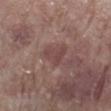The lesion was tiled from a total-body skin photograph and was not biopsied.
This is a white-light tile.
Approximately 3 mm at its widest.
An algorithmic analysis of the crop reported a footprint of about 5 mm², an eccentricity of roughly 0.45, and two-axis asymmetry of about 0.55. And it measured a border-irregularity rating of about 5/10, internal color variation of about 2 on a 0–10 scale, and a peripheral color-asymmetry measure near 0.5. It also reported a nevus-likeness score of about 0/100.
Located on the right lower leg.
A 15 mm crop from a total-body photograph taken for skin-cancer surveillance.
A male subject aged 68 to 72.